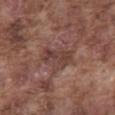<case>
  <biopsy_status>not biopsied; imaged during a skin examination</biopsy_status>
  <image>
    <source>total-body photography crop</source>
    <field_of_view_mm>15</field_of_view_mm>
  </image>
  <lesion_size>
    <long_diameter_mm_approx>4.5</long_diameter_mm_approx>
  </lesion_size>
  <automated_metrics>
    <area_mm2_approx>10.0</area_mm2_approx>
    <eccentricity>0.75</eccentricity>
    <shape_asymmetry>0.25</shape_asymmetry>
    <vs_skin_darker_L>8.0</vs_skin_darker_L>
    <vs_skin_contrast_norm>6.5</vs_skin_contrast_norm>
    <border_irregularity_0_10>3.0</border_irregularity_0_10>
    <color_variation_0_10>4.0</color_variation_0_10>
    <peripheral_color_asymmetry>1.5</peripheral_color_asymmetry>
    <lesion_detection_confidence_0_100>80</lesion_detection_confidence_0_100>
  </automated_metrics>
  <lighting>white-light</lighting>
  <site>front of the torso</site>
  <patient>
    <sex>male</sex>
    <age_approx>75</age_approx>
  </patient>
</case>